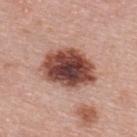• follow-up · total-body-photography surveillance lesion; no biopsy
• image source · total-body-photography crop, ~15 mm field of view
• patient · female, approximately 50 years of age
• automated lesion analysis · border irregularity of about 2 on a 0–10 scale, a color-variation rating of about 8/10, and radial color variation of about 2
• illumination · white-light illumination
• size · ~6.5 mm (longest diameter)
• location · the back